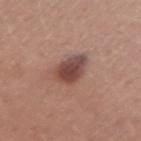No biopsy was performed on this lesion — it was imaged during a full skin examination and was not determined to be concerning. A 15 mm close-up tile from a total-body photography series done for melanoma screening. The patient is a female aged 33–37. Located on the right upper arm.